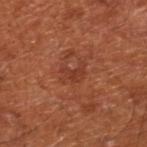The lesion was photographed on a routine skin check and not biopsied; there is no pathology result. Approximately 3 mm at its widest. The lesion is located on the right lower leg. A patient aged approximately 65. The tile uses cross-polarized illumination. Cropped from a total-body skin-imaging series; the visible field is about 15 mm.